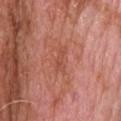{
  "biopsy_status": "not biopsied; imaged during a skin examination"
}